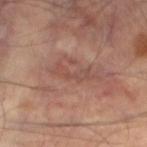Assessment:
The lesion was photographed on a routine skin check and not biopsied; there is no pathology result.
Background:
Longest diameter approximately 4.5 mm. The lesion is located on the left leg. A male patient, roughly 50 years of age. A region of skin cropped from a whole-body photographic capture, roughly 15 mm wide.